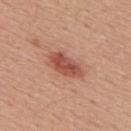  biopsy_status: not biopsied; imaged during a skin examination
  lesion_size:
    long_diameter_mm_approx: 4.5
  site: back
  patient:
    sex: male
    age_approx: 55
  image:
    source: total-body photography crop
    field_of_view_mm: 15